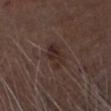Clinical impression: No biopsy was performed on this lesion — it was imaged during a full skin examination and was not determined to be concerning. Background: The tile uses white-light illumination. Approximately 3.5 mm at its widest. Cropped from a total-body skin-imaging series; the visible field is about 15 mm. On the head or neck. The patient is a male in their mid- to late 70s.